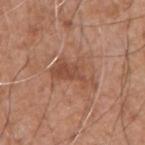Assessment:
Recorded during total-body skin imaging; not selected for excision or biopsy.
Image and clinical context:
The lesion's longest dimension is about 5.5 mm. A 15 mm crop from a total-body photograph taken for skin-cancer surveillance. The total-body-photography lesion software estimated an eccentricity of roughly 0.85 and a symmetry-axis asymmetry near 0.45. It also reported an average lesion color of about L≈49 a*≈23 b*≈31 (CIELAB) and about 8 CIELAB-L* units darker than the surrounding skin. Captured under white-light illumination. Located on the arm. A male patient in their mid- to late 70s.